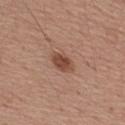This lesion was catalogued during total-body skin photography and was not selected for biopsy. Located on the back. A 15 mm crop from a total-body photograph taken for skin-cancer surveillance. The recorded lesion diameter is about 3 mm. The patient is a male aged 53 to 57.